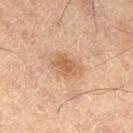follow-up: no biopsy performed (imaged during a skin exam)